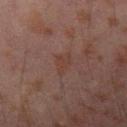The lesion was tiled from a total-body skin photograph and was not biopsied. The patient is a male aged 28–32. From the arm. Longest diameter approximately 3.5 mm. A 15 mm close-up tile from a total-body photography series done for melanoma screening. Imaged with cross-polarized lighting.